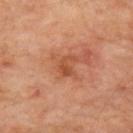biopsy status: imaged on a skin check; not biopsied | subject: male, aged approximately 65 | image: ~15 mm crop, total-body skin-cancer survey | location: the right upper arm | tile lighting: cross-polarized | lesion size: ~2.5 mm (longest diameter).